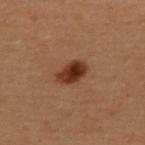  biopsy_status: not biopsied; imaged during a skin examination
  lesion_size:
    long_diameter_mm_approx: 3.0
  image:
    source: total-body photography crop
    field_of_view_mm: 15
  automated_metrics:
    cielab_L: 29
    cielab_a: 20
    cielab_b: 28
    vs_skin_darker_L: 12.0
  patient:
    sex: female
    age_approx: 50
  lighting: cross-polarized
  site: back